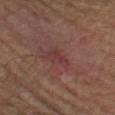  biopsy_status: not biopsied; imaged during a skin examination